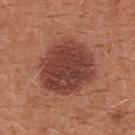{
  "biopsy_status": "not biopsied; imaged during a skin examination",
  "image": {
    "source": "total-body photography crop",
    "field_of_view_mm": 15
  },
  "site": "chest",
  "lighting": "white-light",
  "automated_metrics": {
    "cielab_L": 41,
    "cielab_a": 26,
    "cielab_b": 26,
    "vs_skin_darker_L": 12.0,
    "vs_skin_contrast_norm": 10.0,
    "nevus_likeness_0_100": 90
  },
  "patient": {
    "sex": "female",
    "age_approx": 35
  }
}